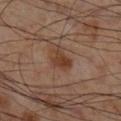Notes:
– workup — imaged on a skin check; not biopsied
– lesion size — about 3 mm
– lighting — cross-polarized
– body site — the right lower leg
– image source — 15 mm crop, total-body photography
– subject — male, aged 53 to 57